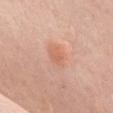Part of a total-body skin-imaging series; this lesion was reviewed on a skin check and was not flagged for biopsy. A female patient aged approximately 50. Approximately 2.5 mm at its widest. From the front of the torso. The tile uses white-light illumination. A roughly 15 mm field-of-view crop from a total-body skin photograph.